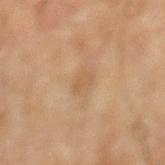The patient is aged 53 to 57.
Automated image analysis of the tile measured a shape-asymmetry score of about 0.3 (0 = symmetric). It also reported a lesion color around L≈57 a*≈18 b*≈36 in CIELAB and roughly 6 lightness units darker than nearby skin. It also reported a border-irregularity rating of about 2.5/10 and a color-variation rating of about 2/10.
On the leg.
The tile uses cross-polarized illumination.
This image is a 15 mm lesion crop taken from a total-body photograph.
Approximately 2.5 mm at its widest.